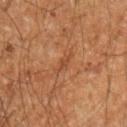Captured during whole-body skin photography for melanoma surveillance; the lesion was not biopsied.
The lesion is on the left upper arm.
The patient is a male in their 60s.
A region of skin cropped from a whole-body photographic capture, roughly 15 mm wide.
The lesion's longest dimension is about 2.5 mm.